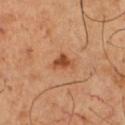Findings:
- acquisition — ~15 mm crop, total-body skin-cancer survey
- illumination — cross-polarized
- subject — male, aged 48 to 52
- site — the chest
- lesion size — ≈2.5 mm